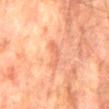biopsy_status: not biopsied; imaged during a skin examination